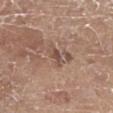The recorded lesion diameter is about 2.5 mm. The lesion is on the left lower leg. Cropped from a total-body skin-imaging series; the visible field is about 15 mm. The patient is a female in their mid- to late 70s.